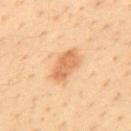Captured during whole-body skin photography for melanoma surveillance; the lesion was not biopsied. Cropped from a total-body skin-imaging series; the visible field is about 15 mm. The lesion is located on the upper back. The subject is a male roughly 35 years of age.